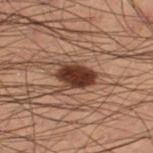workup: imaged on a skin check; not biopsied | subject: male, in their mid-50s | tile lighting: cross-polarized illumination | body site: the right thigh | acquisition: ~15 mm tile from a whole-body skin photo | automated metrics: a footprint of about 7 mm², an outline eccentricity of about 0.85 (0 = round, 1 = elongated), and a shape-asymmetry score of about 0.15 (0 = symmetric); border irregularity of about 1.5 on a 0–10 scale, internal color variation of about 3.5 on a 0–10 scale, and peripheral color asymmetry of about 1.